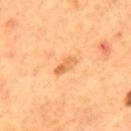Clinical impression: Part of a total-body skin-imaging series; this lesion was reviewed on a skin check and was not flagged for biopsy. Acquisition and patient details: The patient is a male approximately 55 years of age. This is a cross-polarized tile. Cropped from a whole-body photographic skin survey; the tile spans about 15 mm. The lesion is on the mid back. About 3 mm across. An algorithmic analysis of the crop reported an area of roughly 3.5 mm² and a shape-asymmetry score of about 0.2 (0 = symmetric). The analysis additionally found a mean CIELAB color near L≈62 a*≈25 b*≈44, roughly 9 lightness units darker than nearby skin, and a lesion-to-skin contrast of about 6.5 (normalized; higher = more distinct). The analysis additionally found a border-irregularity rating of about 2.5/10.